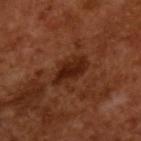No biopsy was performed on this lesion — it was imaged during a full skin examination and was not determined to be concerning. A male subject in their mid-60s. A close-up tile cropped from a whole-body skin photograph, about 15 mm across.